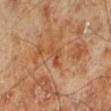Q: What did automated image analysis measure?
A: a lesion area of about 3.5 mm², a shape eccentricity near 0.9, and a shape-asymmetry score of about 0.5 (0 = symmetric); a classifier nevus-likeness of about 0/100 and a lesion-detection confidence of about 100/100
Q: What is the lesion's diameter?
A: ~3.5 mm (longest diameter)
Q: What kind of image is this?
A: total-body-photography crop, ~15 mm field of view
Q: Lesion location?
A: the leg
Q: What are the patient's age and sex?
A: male, aged around 70
Q: What lighting was used for the tile?
A: cross-polarized illumination